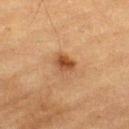Clinical impression:
Recorded during total-body skin imaging; not selected for excision or biopsy.
Background:
Located on the left thigh. Approximately 2.5 mm at its widest. The total-body-photography lesion software estimated a color-variation rating of about 4.5/10 and a peripheral color-asymmetry measure near 1.5. A roughly 15 mm field-of-view crop from a total-body skin photograph. A male patient, approximately 85 years of age or older.